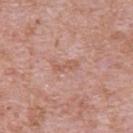Findings:
- anatomic site — the upper back
- imaging modality — total-body-photography crop, ~15 mm field of view
- patient — male, aged 48–52